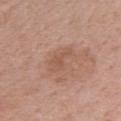The tile uses white-light illumination.
A male subject approximately 55 years of age.
The lesion is on the front of the torso.
The recorded lesion diameter is about 3.5 mm.
This image is a 15 mm lesion crop taken from a total-body photograph.